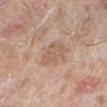Clinical impression:
No biopsy was performed on this lesion — it was imaged during a full skin examination and was not determined to be concerning.
Context:
This is a white-light tile. A male patient aged 78–82. The lesion is on the left lower leg. A 15 mm crop from a total-body photograph taken for skin-cancer surveillance. An algorithmic analysis of the crop reported an area of roughly 7 mm², an outline eccentricity of about 0.5 (0 = round, 1 = elongated), and two-axis asymmetry of about 0.3. The software also gave a classifier nevus-likeness of about 0/100 and lesion-presence confidence of about 100/100. The recorded lesion diameter is about 3 mm.